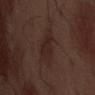Notes:
* biopsy status — no biopsy performed (imaged during a skin exam)
* subject — male, aged 68 to 72
* automated metrics — an automated nevus-likeness rating near 65 out of 100 and lesion-presence confidence of about 100/100
* site — the abdomen
* imaging modality — total-body-photography crop, ~15 mm field of view
* tile lighting — white-light
* size — about 5 mm